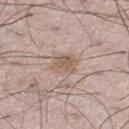This lesion was catalogued during total-body skin photography and was not selected for biopsy. The lesion is on the right thigh. A male patient, approximately 50 years of age. A 15 mm crop from a total-body photograph taken for skin-cancer surveillance.